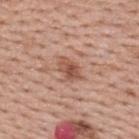workup: total-body-photography surveillance lesion; no biopsy | image: ~15 mm tile from a whole-body skin photo | location: the upper back | patient: male, aged around 40 | size: about 3.5 mm | lighting: white-light illumination | automated lesion analysis: a lesion area of about 5.5 mm², a shape eccentricity near 0.75, and two-axis asymmetry of about 0.2; a lesion–skin lightness drop of about 11 and a normalized lesion–skin contrast near 7.5; a border-irregularity rating of about 2/10 and a within-lesion color-variation index near 4/10; a nevus-likeness score of about 70/100 and a detector confidence of about 100 out of 100 that the crop contains a lesion.